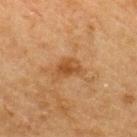workup = no biopsy performed (imaged during a skin exam) | subject = male, approximately 50 years of age | illumination = cross-polarized illumination | anatomic site = the upper back | lesion size = ~3 mm (longest diameter) | image source = ~15 mm tile from a whole-body skin photo.